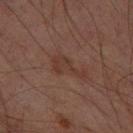The lesion is on the right thigh.
About 4.5 mm across.
The tile uses cross-polarized illumination.
A roughly 15 mm field-of-view crop from a total-body skin photograph.
A male subject aged approximately 60.